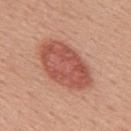  biopsy_status: not biopsied; imaged during a skin examination
  site: upper back
  patient:
    sex: male
    age_approx: 55
  image:
    source: total-body photography crop
    field_of_view_mm: 15